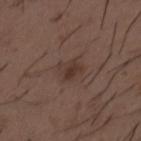No biopsy was performed on this lesion — it was imaged during a full skin examination and was not determined to be concerning.